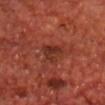{"biopsy_status": "not biopsied; imaged during a skin examination", "site": "chest", "image": {"source": "total-body photography crop", "field_of_view_mm": 15}, "patient": {"sex": "male", "age_approx": 70}}